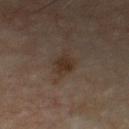Q: Was a biopsy performed?
A: imaged on a skin check; not biopsied
Q: What did automated image analysis measure?
A: a lesion area of about 4.5 mm² and two-axis asymmetry of about 0.3; a lesion color around L≈28 a*≈13 b*≈21 in CIELAB and about 7 CIELAB-L* units darker than the surrounding skin; internal color variation of about 2.5 on a 0–10 scale and a peripheral color-asymmetry measure near 1
Q: What is the imaging modality?
A: total-body-photography crop, ~15 mm field of view
Q: Who is the patient?
A: male, aged 53 to 57
Q: Lesion size?
A: ~2.5 mm (longest diameter)
Q: How was the tile lit?
A: cross-polarized illumination
Q: Lesion location?
A: the chest